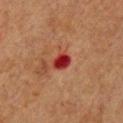Recorded during total-body skin imaging; not selected for excision or biopsy.
From the chest.
Automated tile analysis of the lesion measured a footprint of about 4.5 mm² and an outline eccentricity of about 0.65 (0 = round, 1 = elongated). The software also gave a mean CIELAB color near L≈33 a*≈36 b*≈29 and a lesion-to-skin contrast of about 11.5 (normalized; higher = more distinct). The software also gave a border-irregularity index near 1.5/10, a color-variation rating of about 4/10, and a peripheral color-asymmetry measure near 1.5.
A 15 mm crop from a total-body photograph taken for skin-cancer surveillance.
This is a cross-polarized tile.
A female subject aged 38–42.